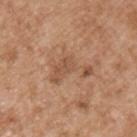The lesion was photographed on a routine skin check and not biopsied; there is no pathology result.
A male subject aged 53 to 57.
From the left upper arm.
The tile uses white-light illumination.
Cropped from a total-body skin-imaging series; the visible field is about 15 mm.
Approximately 5.5 mm at its widest.
The lesion-visualizer software estimated two-axis asymmetry of about 0.65. The software also gave roughly 8 lightness units darker than nearby skin and a lesion-to-skin contrast of about 5.5 (normalized; higher = more distinct). The software also gave border irregularity of about 8.5 on a 0–10 scale, internal color variation of about 3 on a 0–10 scale, and a peripheral color-asymmetry measure near 1.